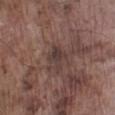biopsy status = total-body-photography surveillance lesion; no biopsy | tile lighting = white-light illumination | diameter = about 2.5 mm | subject = male, about 75 years old | image source = total-body-photography crop, ~15 mm field of view | body site = the left lower leg.